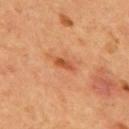biopsy_status: not biopsied; imaged during a skin examination
image:
  source: total-body photography crop
  field_of_view_mm: 15
lesion_size:
  long_diameter_mm_approx: 2.5
automated_metrics:
  cielab_L: 48
  cielab_a: 28
  cielab_b: 38
  vs_skin_darker_L: 10.0
  vs_skin_contrast_norm: 7.5
patient:
  sex: male
  age_approx: 55
site: mid back
lighting: cross-polarized